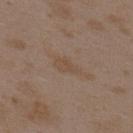Notes:
- workup — catalogued during a skin exam; not biopsied
- image source — total-body-photography crop, ~15 mm field of view
- subject — female, about 35 years old
- lighting — white-light illumination
- lesion size — ~3.5 mm (longest diameter)
- location — the upper back